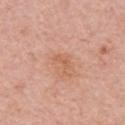notes = catalogued during a skin exam; not biopsied | patient = female, about 65 years old | image = 15 mm crop, total-body photography | location = the left upper arm.